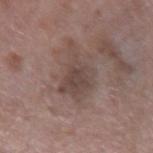patient: male, aged around 60
image source: total-body-photography crop, ~15 mm field of view
location: the right forearm